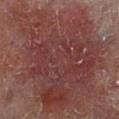Q: Is there a histopathology result?
A: catalogued during a skin exam; not biopsied
Q: Lesion size?
A: ~16 mm (longest diameter)
Q: What are the patient's age and sex?
A: male, aged around 60
Q: How was the tile lit?
A: cross-polarized
Q: What did automated image analysis measure?
A: a border-irregularity rating of about 7/10, internal color variation of about 5 on a 0–10 scale, and radial color variation of about 1.5
Q: What kind of image is this?
A: total-body-photography crop, ~15 mm field of view
Q: Where on the body is the lesion?
A: the right lower leg